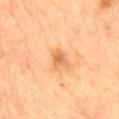Part of a total-body skin-imaging series; this lesion was reviewed on a skin check and was not flagged for biopsy. Cropped from a total-body skin-imaging series; the visible field is about 15 mm. A male subject, aged around 85 or older. On the mid back.